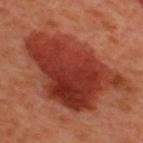follow-up=no biopsy performed (imaged during a skin exam); patient=male, approximately 50 years of age; image=~15 mm tile from a whole-body skin photo; anatomic site=the upper back.